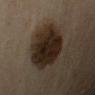Part of a total-body skin-imaging series; this lesion was reviewed on a skin check and was not flagged for biopsy. Located on the right upper arm. A male subject aged 53–57. A 15 mm close-up tile from a total-body photography series done for melanoma screening.